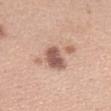Q: Was a biopsy performed?
A: catalogued during a skin exam; not biopsied
Q: How large is the lesion?
A: about 5.5 mm
Q: What is the anatomic site?
A: the left upper arm
Q: How was this image acquired?
A: total-body-photography crop, ~15 mm field of view
Q: Who is the patient?
A: female, aged 28–32